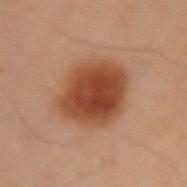This lesion was catalogued during total-body skin photography and was not selected for biopsy. A 15 mm close-up extracted from a 3D total-body photography capture. The lesion's longest dimension is about 5.5 mm. Automated tile analysis of the lesion measured a mean CIELAB color near L≈36 a*≈20 b*≈28 and about 12 CIELAB-L* units darker than the surrounding skin. The software also gave a color-variation rating of about 4.5/10 and peripheral color asymmetry of about 1.5. It also reported a classifier nevus-likeness of about 100/100 and lesion-presence confidence of about 100/100. Captured under cross-polarized illumination. From the left upper arm. A male subject in their mid-50s.